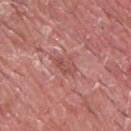Acquisition and patient details: The lesion is located on the front of the torso. This image is a 15 mm lesion crop taken from a total-body photograph. The tile uses white-light illumination. A male subject, aged 38–42. The lesion's longest dimension is about 3.5 mm.